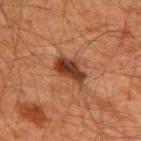notes: imaged on a skin check; not biopsied
lighting: cross-polarized
anatomic site: the back
subject: male, roughly 60 years of age
size: ~4 mm (longest diameter)
image: total-body-photography crop, ~15 mm field of view